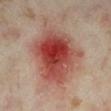Impression:
The lesion was tiled from a total-body skin photograph and was not biopsied.
Background:
About 7 mm across. A female subject, approximately 35 years of age. An algorithmic analysis of the crop reported border irregularity of about 2.5 on a 0–10 scale and peripheral color asymmetry of about 3.5. The analysis additionally found a nevus-likeness score of about 0/100. A lesion tile, about 15 mm wide, cut from a 3D total-body photograph. The tile uses cross-polarized illumination. On the left lower leg.